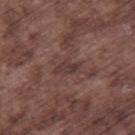Imaged during a routine full-body skin examination; the lesion was not biopsied and no histopathology is available.
Cropped from a whole-body photographic skin survey; the tile spans about 15 mm.
A male subject, aged 73 to 77.
Longest diameter approximately 3 mm.
The tile uses white-light illumination.
The lesion is located on the right thigh.